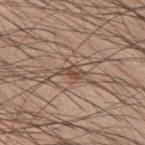Imaged during a routine full-body skin examination; the lesion was not biopsied and no histopathology is available. A male patient aged approximately 60. The lesion-visualizer software estimated a lesion–skin lightness drop of about 10 and a normalized border contrast of about 7.5. And it measured border irregularity of about 3.5 on a 0–10 scale, a within-lesion color-variation index near 1.5/10, and a peripheral color-asymmetry measure near 0.5. And it measured a lesion-detection confidence of about 90/100. Cropped from a whole-body photographic skin survey; the tile spans about 15 mm. Located on the upper back.